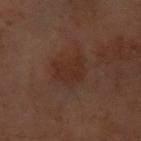Clinical impression:
Imaged during a routine full-body skin examination; the lesion was not biopsied and no histopathology is available.
Image and clinical context:
A female subject aged approximately 60. The tile uses cross-polarized illumination. The lesion is on the front of the torso. A 15 mm close-up tile from a total-body photography series done for melanoma screening.